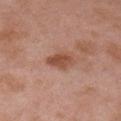Acquisition and patient details:
From the right upper arm. A male patient, roughly 55 years of age. The tile uses white-light illumination. A lesion tile, about 15 mm wide, cut from a 3D total-body photograph.